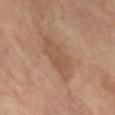  patient:
    sex: female
    age_approx: 80
  site: right leg
  image:
    source: total-body photography crop
    field_of_view_mm: 15
  lighting: cross-polarized
  lesion_size:
    long_diameter_mm_approx: 6.5
  automated_metrics:
    cielab_L: 46
    cielab_a: 18
    cielab_b: 27
    vs_skin_darker_L: 7.0
    border_irregularity_0_10: 4.0
    color_variation_0_10: 2.5
    peripheral_color_asymmetry: 1.0
    nevus_likeness_0_100: 0
    lesion_detection_confidence_0_100: 100